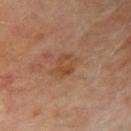follow-up: no biopsy performed (imaged during a skin exam)
imaging modality: total-body-photography crop, ~15 mm field of view
body site: the arm
size: ≈3.5 mm
subject: male, about 65 years old
tile lighting: cross-polarized illumination